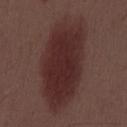Q: Is there a histopathology result?
A: no biopsy performed (imaged during a skin exam)
Q: Illumination type?
A: white-light illumination
Q: What is the lesion's diameter?
A: ~11.5 mm (longest diameter)
Q: How was this image acquired?
A: total-body-photography crop, ~15 mm field of view
Q: What are the patient's age and sex?
A: male, aged around 55
Q: Where on the body is the lesion?
A: the mid back